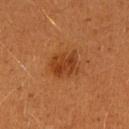| field | value |
|---|---|
| workup | catalogued during a skin exam; not biopsied |
| patient | female, aged approximately 30 |
| site | the arm |
| automated metrics | a mean CIELAB color near L≈37 a*≈25 b*≈37, about 8 CIELAB-L* units darker than the surrounding skin, and a normalized lesion–skin contrast near 7 |
| tile lighting | cross-polarized |
| image source | 15 mm crop, total-body photography |